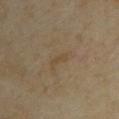Impression:
This lesion was catalogued during total-body skin photography and was not selected for biopsy.
Background:
The tile uses cross-polarized illumination. A 15 mm crop from a total-body photograph taken for skin-cancer surveillance. From the upper back. The recorded lesion diameter is about 2.5 mm. The patient is a female approximately 35 years of age. The total-body-photography lesion software estimated an area of roughly 2.5 mm² and an outline eccentricity of about 0.9 (0 = round, 1 = elongated). And it measured a mean CIELAB color near L≈43 a*≈11 b*≈29. And it measured internal color variation of about 0 on a 0–10 scale and peripheral color asymmetry of about 0. The software also gave a classifier nevus-likeness of about 0/100.